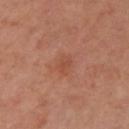Clinical impression:
Imaged during a routine full-body skin examination; the lesion was not biopsied and no histopathology is available.
Image and clinical context:
Located on the left upper arm. The patient is a female aged 58 to 62. A lesion tile, about 15 mm wide, cut from a 3D total-body photograph. The total-body-photography lesion software estimated a mean CIELAB color near L≈50 a*≈26 b*≈32, about 6 CIELAB-L* units darker than the surrounding skin, and a normalized border contrast of about 5. The analysis additionally found border irregularity of about 2.5 on a 0–10 scale and internal color variation of about 1 on a 0–10 scale. The software also gave a classifier nevus-likeness of about 0/100. Captured under cross-polarized illumination.